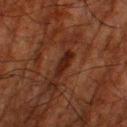workup — catalogued during a skin exam; not biopsied | subject — male, aged 78–82 | illumination — cross-polarized illumination | location — the left thigh | lesion size — ~3.5 mm (longest diameter) | image — total-body-photography crop, ~15 mm field of view | automated lesion analysis — a lesion area of about 4 mm², a shape eccentricity near 0.9, and a shape-asymmetry score of about 0.3 (0 = symmetric); an average lesion color of about L≈18 a*≈19 b*≈21 (CIELAB) and a lesion-to-skin contrast of about 9.5 (normalized; higher = more distinct); a border-irregularity rating of about 3.5/10, a within-lesion color-variation index near 1.5/10, and peripheral color asymmetry of about 0.5; an automated nevus-likeness rating near 30 out of 100.